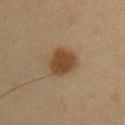Part of a total-body skin-imaging series; this lesion was reviewed on a skin check and was not flagged for biopsy.